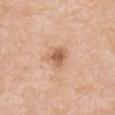follow-up — total-body-photography surveillance lesion; no biopsy | subject — male, aged 78–82 | site — the arm | automated lesion analysis — a lesion color around L≈59 a*≈22 b*≈34 in CIELAB, a lesion–skin lightness drop of about 12, and a normalized lesion–skin contrast near 8; a border-irregularity index near 3/10, a color-variation rating of about 3/10, and a peripheral color-asymmetry measure near 1 | tile lighting — white-light illumination | diameter — ≈2.5 mm | acquisition — ~15 mm tile from a whole-body skin photo.